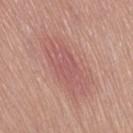anatomic site = the left thigh
diameter = ~5 mm (longest diameter)
TBP lesion metrics = a lesion color around L≈55 a*≈26 b*≈23 in CIELAB, about 6 CIELAB-L* units darker than the surrounding skin, and a normalized border contrast of about 4.5; a border-irregularity index near 3.5/10 and a peripheral color-asymmetry measure near 0.5
image source = 15 mm crop, total-body photography
patient = female, in their mid-60s